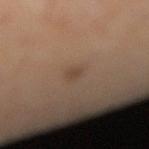Clinical impression: The lesion was photographed on a routine skin check and not biopsied; there is no pathology result. Background: Located on the right lower leg. A 15 mm close-up tile from a total-body photography series done for melanoma screening. A female patient about 35 years old.